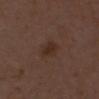biopsy status: no biopsy performed (imaged during a skin exam); image: ~15 mm tile from a whole-body skin photo; subject: female, approximately 50 years of age; location: the front of the torso; diameter: about 3 mm.